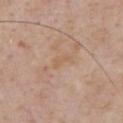Impression:
No biopsy was performed on this lesion — it was imaged during a full skin examination and was not determined to be concerning.
Image and clinical context:
A 15 mm close-up extracted from a 3D total-body photography capture. The subject is a male aged 58–62. On the front of the torso.